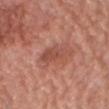The lesion was photographed on a routine skin check and not biopsied; there is no pathology result.
A 15 mm close-up tile from a total-body photography series done for melanoma screening.
Located on the front of the torso.
A male patient aged approximately 80.